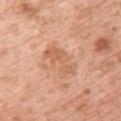workup: total-body-photography surveillance lesion; no biopsy.